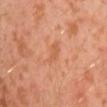Q: Was a biopsy performed?
A: catalogued during a skin exam; not biopsied
Q: Lesion location?
A: the left arm
Q: What are the patient's age and sex?
A: male, in their 30s
Q: What kind of image is this?
A: 15 mm crop, total-body photography